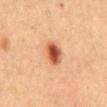The lesion is located on the mid back.
The patient is a female in their 60s.
Captured under cross-polarized illumination.
A close-up tile cropped from a whole-body skin photograph, about 15 mm across.
Approximately 3.5 mm at its widest.